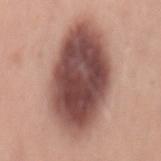<lesion>
<biopsy_status>not biopsied; imaged during a skin examination</biopsy_status>
<image>
  <source>total-body photography crop</source>
  <field_of_view_mm>15</field_of_view_mm>
</image>
<lighting>white-light</lighting>
<site>mid back</site>
<lesion_size>
  <long_diameter_mm_approx>11.5</long_diameter_mm_approx>
</lesion_size>
<patient>
  <sex>female</sex>
  <age_approx>40</age_approx>
</patient>
</lesion>